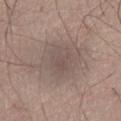follow-up = imaged on a skin check; not biopsied | TBP lesion metrics = a mean CIELAB color near L≈52 a*≈13 b*≈20, roughly 7 lightness units darker than nearby skin, and a normalized lesion–skin contrast near 5.5; a within-lesion color-variation index near 2.5/10 and radial color variation of about 1; a nevus-likeness score of about 0/100 | acquisition = total-body-photography crop, ~15 mm field of view | size = ~7 mm (longest diameter) | subject = male, aged 58–62 | lighting = white-light | site = the abdomen.